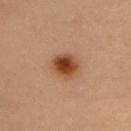Clinical impression:
This lesion was catalogued during total-body skin photography and was not selected for biopsy.